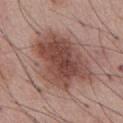Q: Was this lesion biopsied?
A: total-body-photography surveillance lesion; no biopsy
Q: Who is the patient?
A: male, about 55 years old
Q: What is the imaging modality?
A: ~15 mm crop, total-body skin-cancer survey
Q: Where on the body is the lesion?
A: the abdomen
Q: What lighting was used for the tile?
A: white-light